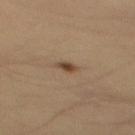Impression: This lesion was catalogued during total-body skin photography and was not selected for biopsy. Background: Cropped from a whole-body photographic skin survey; the tile spans about 15 mm. The lesion is located on the mid back. About 2 mm across. The lesion-visualizer software estimated a border-irregularity index near 3/10, internal color variation of about 3 on a 0–10 scale, and peripheral color asymmetry of about 1. The software also gave a classifier nevus-likeness of about 95/100 and a detector confidence of about 100 out of 100 that the crop contains a lesion. A male subject in their mid-30s.